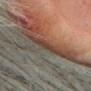A 15 mm close-up extracted from a 3D total-body photography capture. Imaged with cross-polarized lighting. The lesion is located on the head or neck. Longest diameter approximately 3 mm. The total-body-photography lesion software estimated a normalized border contrast of about 6.5. A female patient about 75 years old. Histopathological examination showed an actinic keratosis — a lesion of indeterminate malignant potential.